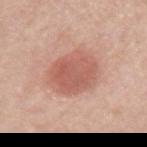Q: Where on the body is the lesion?
A: the left upper arm
Q: What are the patient's age and sex?
A: male, in their mid- to late 50s
Q: What kind of image is this?
A: total-body-photography crop, ~15 mm field of view
Q: Automated lesion metrics?
A: a footprint of about 20 mm², an outline eccentricity of about 0.6 (0 = round, 1 = elongated), and two-axis asymmetry of about 0.1; an automated nevus-likeness rating near 90 out of 100 and a detector confidence of about 100 out of 100 that the crop contains a lesion
Q: What is the lesion's diameter?
A: about 5.5 mm